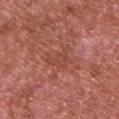The lesion was tiled from a total-body skin photograph and was not biopsied. A roughly 15 mm field-of-view crop from a total-body skin photograph. A male patient about 65 years old. The lesion is located on the back.